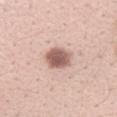{
  "biopsy_status": "not biopsied; imaged during a skin examination",
  "patient": {
    "sex": "female",
    "age_approx": 40
  },
  "lighting": "white-light",
  "image": {
    "source": "total-body photography crop",
    "field_of_view_mm": 15
  },
  "lesion_size": {
    "long_diameter_mm_approx": 3.5
  },
  "site": "left forearm"
}